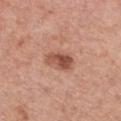Q: Was a biopsy performed?
A: total-body-photography surveillance lesion; no biopsy
Q: What kind of image is this?
A: ~15 mm crop, total-body skin-cancer survey
Q: Lesion location?
A: the chest
Q: Who is the patient?
A: female, aged 73–77